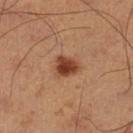{"biopsy_status": "not biopsied; imaged during a skin examination", "lesion_size": {"long_diameter_mm_approx": 3.0}, "site": "left lower leg", "lighting": "cross-polarized", "image": {"source": "total-body photography crop", "field_of_view_mm": 15}, "patient": {"sex": "male", "age_approx": 60}, "automated_metrics": {"cielab_L": 42, "cielab_a": 23, "cielab_b": 32, "vs_skin_darker_L": 13.0, "vs_skin_contrast_norm": 10.0, "border_irregularity_0_10": 2.0, "color_variation_0_10": 4.0, "peripheral_color_asymmetry": 1.5}}